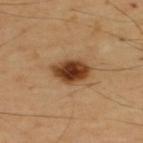Acquisition and patient details: A male patient, aged 63 to 67. The lesion is on the upper back. Cropped from a whole-body photographic skin survey; the tile spans about 15 mm.